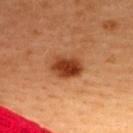Imaged with cross-polarized lighting. A female subject in their mid-30s. On the upper back. Measured at roughly 4 mm in maximum diameter. A 15 mm close-up tile from a total-body photography series done for melanoma screening.